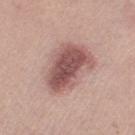Case summary:
• biopsy status: no biopsy performed (imaged during a skin exam)
• lighting: white-light
• TBP lesion metrics: a classifier nevus-likeness of about 65/100 and a lesion-detection confidence of about 100/100
• patient: female, aged around 20
• image source: ~15 mm crop, total-body skin-cancer survey
• anatomic site: the left thigh